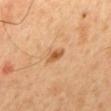The lesion was tiled from a total-body skin photograph and was not biopsied. The lesion's longest dimension is about 3 mm. From the mid back. A close-up tile cropped from a whole-body skin photograph, about 15 mm across. Captured under cross-polarized illumination. A male patient, aged 43 to 47.